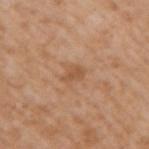The lesion was photographed on a routine skin check and not biopsied; there is no pathology result.